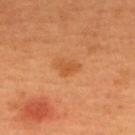{"automated_metrics": {"area_mm2_approx": 3.5, "eccentricity": 0.8, "shape_asymmetry": 0.45, "cielab_L": 54, "cielab_a": 28, "cielab_b": 44, "vs_skin_darker_L": 8.0, "vs_skin_contrast_norm": 6.0}, "patient": {"sex": "female", "age_approx": 50}, "lesion_size": {"long_diameter_mm_approx": 2.5}, "image": {"source": "total-body photography crop", "field_of_view_mm": 15}}